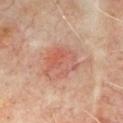No biopsy was performed on this lesion — it was imaged during a full skin examination and was not determined to be concerning. From the chest. Imaged with cross-polarized lighting. The patient is roughly 65 years of age. The lesion's longest dimension is about 4.5 mm. The total-body-photography lesion software estimated a mean CIELAB color near L≈57 a*≈26 b*≈29, about 8 CIELAB-L* units darker than the surrounding skin, and a normalized border contrast of about 5.5. The analysis additionally found a color-variation rating of about 6.5/10 and a peripheral color-asymmetry measure near 2. A roughly 15 mm field-of-view crop from a total-body skin photograph.